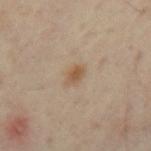Clinical impression:
This lesion was catalogued during total-body skin photography and was not selected for biopsy.
Clinical summary:
From the arm. Cropped from a total-body skin-imaging series; the visible field is about 15 mm. The patient is a male aged around 45. Captured under cross-polarized illumination.